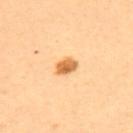The lesion was photographed on a routine skin check and not biopsied; there is no pathology result.
Imaged with cross-polarized lighting.
The total-body-photography lesion software estimated a footprint of about 4.5 mm², a shape eccentricity near 0.75, and two-axis asymmetry of about 0.2. The software also gave a lesion color around L≈66 a*≈24 b*≈46 in CIELAB. The software also gave a border-irregularity index near 1.5/10 and a within-lesion color-variation index near 4/10.
The lesion is on the back.
A female patient, aged approximately 35.
A 15 mm crop from a total-body photograph taken for skin-cancer surveillance.
The lesion's longest dimension is about 3 mm.